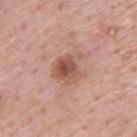Captured during whole-body skin photography for melanoma surveillance; the lesion was not biopsied.
About 4 mm across.
This image is a 15 mm lesion crop taken from a total-body photograph.
This is a white-light tile.
Located on the upper back.
An algorithmic analysis of the crop reported a border-irregularity index near 3.5/10, internal color variation of about 6.5 on a 0–10 scale, and a peripheral color-asymmetry measure near 2. It also reported an automated nevus-likeness rating near 30 out of 100 and a lesion-detection confidence of about 100/100.
A male patient approximately 75 years of age.